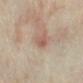Findings:
• workup · total-body-photography surveillance lesion; no biopsy
• image source · total-body-photography crop, ~15 mm field of view
• body site · the left forearm
• subject · female, aged 33 to 37